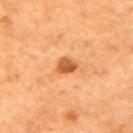biopsy status: imaged on a skin check; not biopsied | body site: the left upper arm | TBP lesion metrics: an eccentricity of roughly 0.45 and two-axis asymmetry of about 0.3; a border-irregularity index near 2.5/10 and a color-variation rating of about 3/10 | subject: female, aged approximately 65 | diameter: ≈2.5 mm | lighting: cross-polarized | imaging modality: 15 mm crop, total-body photography.